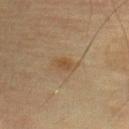Part of a total-body skin-imaging series; this lesion was reviewed on a skin check and was not flagged for biopsy. The lesion-visualizer software estimated an eccentricity of roughly 0.75 and a shape-asymmetry score of about 0.2 (0 = symmetric). And it measured a lesion color around L≈39 a*≈13 b*≈28 in CIELAB, a lesion–skin lightness drop of about 6, and a normalized border contrast of about 6. And it measured a nevus-likeness score of about 15/100 and a detector confidence of about 100 out of 100 that the crop contains a lesion. A female subject, about 55 years old. Imaged with cross-polarized lighting. From the right lower leg. A lesion tile, about 15 mm wide, cut from a 3D total-body photograph.